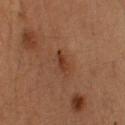location — the head or neck; subject — male, aged around 35; size — ≈2.5 mm; tile lighting — cross-polarized; image source — ~15 mm tile from a whole-body skin photo.